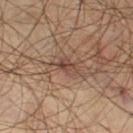Part of a total-body skin-imaging series; this lesion was reviewed on a skin check and was not flagged for biopsy. A male patient in their mid-50s. A 15 mm close-up tile from a total-body photography series done for melanoma screening. The lesion is on the left thigh. The tile uses cross-polarized illumination.